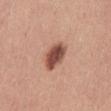| key | value |
|---|---|
| workup | total-body-photography surveillance lesion; no biopsy |
| image | ~15 mm crop, total-body skin-cancer survey |
| lighting | white-light |
| patient | female, roughly 40 years of age |
| site | the abdomen |
| lesion size | ≈3.5 mm |